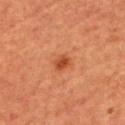Impression: Captured during whole-body skin photography for melanoma surveillance; the lesion was not biopsied. Image and clinical context: The recorded lesion diameter is about 2.5 mm. The lesion is on the front of the torso. A 15 mm close-up extracted from a 3D total-body photography capture. A male subject approximately 65 years of age.